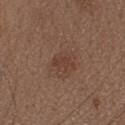Assessment: No biopsy was performed on this lesion — it was imaged during a full skin examination and was not determined to be concerning. Acquisition and patient details: Located on the head or neck. The recorded lesion diameter is about 2.5 mm. A female patient, approximately 30 years of age. A roughly 15 mm field-of-view crop from a total-body skin photograph. An algorithmic analysis of the crop reported a lesion color around L≈39 a*≈19 b*≈26 in CIELAB, roughly 6 lightness units darker than nearby skin, and a normalized border contrast of about 5.5. The analysis additionally found a border-irregularity index near 3.5/10, a within-lesion color-variation index near 1/10, and peripheral color asymmetry of about 0.5.